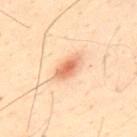<lesion>
  <biopsy_status>not biopsied; imaged during a skin examination</biopsy_status>
  <patient>
    <sex>male</sex>
    <age_approx>50</age_approx>
  </patient>
  <automated_metrics>
    <area_mm2_approx>5.0</area_mm2_approx>
    <eccentricity>0.9</eccentricity>
    <shape_asymmetry>0.2</shape_asymmetry>
    <vs_skin_darker_L>12.0</vs_skin_darker_L>
    <vs_skin_contrast_norm>8.0</vs_skin_contrast_norm>
    <border_irregularity_0_10>2.5</border_irregularity_0_10>
    <color_variation_0_10>3.0</color_variation_0_10>
    <peripheral_color_asymmetry>0.5</peripheral_color_asymmetry>
    <nevus_likeness_0_100>100</nevus_likeness_0_100>
    <lesion_detection_confidence_0_100>100</lesion_detection_confidence_0_100>
  </automated_metrics>
  <image>
    <source>total-body photography crop</source>
    <field_of_view_mm>15</field_of_view_mm>
  </image>
  <site>upper back</site>
</lesion>